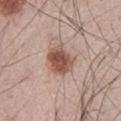The lesion-visualizer software estimated a footprint of about 10 mm². It also reported a nevus-likeness score of about 100/100 and a lesion-detection confidence of about 100/100.
The lesion is located on the abdomen.
The patient is a male aged approximately 65.
The lesion's longest dimension is about 3.5 mm.
A close-up tile cropped from a whole-body skin photograph, about 15 mm across.